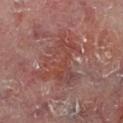The lesion was photographed on a routine skin check and not biopsied; there is no pathology result. About 6 mm across. A lesion tile, about 15 mm wide, cut from a 3D total-body photograph. A male subject, aged 58–62. The tile uses cross-polarized illumination. The lesion is on the right lower leg.